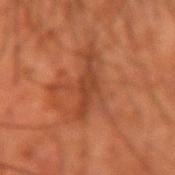{"biopsy_status": "not biopsied; imaged during a skin examination", "site": "arm", "automated_metrics": {"vs_skin_darker_L": 8.0, "vs_skin_contrast_norm": 7.0}, "patient": {"sex": "male", "age_approx": 55}, "image": {"source": "total-body photography crop", "field_of_view_mm": 15}, "lighting": "cross-polarized"}